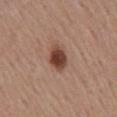Q: What is the anatomic site?
A: the mid back
Q: How was the tile lit?
A: white-light illumination
Q: Lesion size?
A: about 3.5 mm
Q: What are the patient's age and sex?
A: male, in their mid- to late 50s
Q: What is the imaging modality?
A: ~15 mm tile from a whole-body skin photo